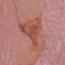Recorded during total-body skin imaging; not selected for excision or biopsy. Located on the left forearm. About 5 mm across. A male subject about 60 years old. A roughly 15 mm field-of-view crop from a total-body skin photograph. Captured under white-light illumination.